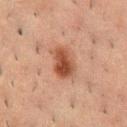– follow-up — imaged on a skin check; not biopsied
– lighting — cross-polarized illumination
– image — ~15 mm tile from a whole-body skin photo
– subject — male, aged 48–52
– automated metrics — a nevus-likeness score of about 100/100 and a detector confidence of about 100 out of 100 that the crop contains a lesion
– size — ~4 mm (longest diameter)
– site — the chest